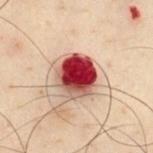notes: no biopsy performed (imaged during a skin exam); image source: ~15 mm tile from a whole-body skin photo; patient: male, aged 48–52; lighting: cross-polarized; site: the chest.